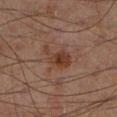Part of a total-body skin-imaging series; this lesion was reviewed on a skin check and was not flagged for biopsy. On the left lower leg. A lesion tile, about 15 mm wide, cut from a 3D total-body photograph. A male patient approximately 65 years of age.